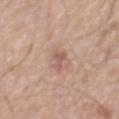A male subject, roughly 55 years of age.
Automated tile analysis of the lesion measured an area of roughly 3 mm², an eccentricity of roughly 0.9, and a symmetry-axis asymmetry near 0.55. The software also gave an average lesion color of about L≈57 a*≈21 b*≈24 (CIELAB) and a lesion–skin lightness drop of about 9.
The lesion is on the left upper arm.
The tile uses white-light illumination.
This image is a 15 mm lesion crop taken from a total-body photograph.